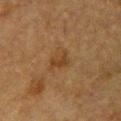Q: Is there a histopathology result?
A: imaged on a skin check; not biopsied
Q: What did automated image analysis measure?
A: an eccentricity of roughly 0.75 and two-axis asymmetry of about 0.4; a border-irregularity rating of about 4/10, a within-lesion color-variation index near 2/10, and radial color variation of about 1; a nevus-likeness score of about 15/100
Q: What is the anatomic site?
A: the chest
Q: What is the lesion's diameter?
A: ≈3 mm
Q: What lighting was used for the tile?
A: cross-polarized
Q: What kind of image is this?
A: total-body-photography crop, ~15 mm field of view
Q: Who is the patient?
A: female, aged approximately 55